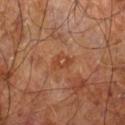Impression: No biopsy was performed on this lesion — it was imaged during a full skin examination and was not determined to be concerning. Context: A lesion tile, about 15 mm wide, cut from a 3D total-body photograph. A male patient, roughly 60 years of age. The tile uses cross-polarized illumination. From the leg.